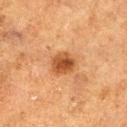  site: leg
  lighting: cross-polarized
  patient:
    sex: male
    age_approx: 75
  image:
    source: total-body photography crop
    field_of_view_mm: 15